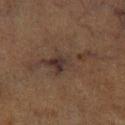biopsy status = imaged on a skin check; not biopsied
illumination = cross-polarized
patient = male, roughly 75 years of age
anatomic site = the left lower leg
image = ~15 mm tile from a whole-body skin photo
size = about 8.5 mm